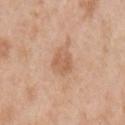Findings:
- biopsy status — imaged on a skin check; not biopsied
- subject — male, aged approximately 50
- automated metrics — a footprint of about 6 mm² and a symmetry-axis asymmetry near 0.2; a border-irregularity index near 2/10, a color-variation rating of about 2/10, and a peripheral color-asymmetry measure near 0.5; a classifier nevus-likeness of about 15/100 and a lesion-detection confidence of about 100/100
- imaging modality — ~15 mm tile from a whole-body skin photo
- location — the arm